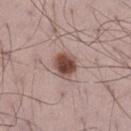Clinical impression: Imaged during a routine full-body skin examination; the lesion was not biopsied and no histopathology is available. Acquisition and patient details: The tile uses white-light illumination. A 15 mm crop from a total-body photograph taken for skin-cancer surveillance. Automated tile analysis of the lesion measured a lesion–skin lightness drop of about 16 and a normalized lesion–skin contrast near 11. And it measured a classifier nevus-likeness of about 100/100 and lesion-presence confidence of about 100/100. The patient is a male aged 68–72. From the left thigh.